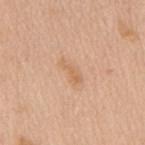Clinical impression: The lesion was photographed on a routine skin check and not biopsied; there is no pathology result. Context: Located on the mid back. The patient is a female about 70 years old. A close-up tile cropped from a whole-body skin photograph, about 15 mm across.